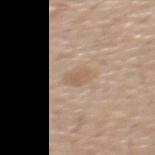biopsy status: catalogued during a skin exam; not biopsied
subject: male, aged around 60
tile lighting: white-light
acquisition: 15 mm crop, total-body photography
anatomic site: the upper back